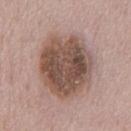Impression: No biopsy was performed on this lesion — it was imaged during a full skin examination and was not determined to be concerning. Context: On the chest. The total-body-photography lesion software estimated an average lesion color of about L≈50 a*≈17 b*≈24 (CIELAB) and a lesion-to-skin contrast of about 10 (normalized; higher = more distinct). The analysis additionally found a border-irregularity index near 1.5/10 and a within-lesion color-variation index near 6/10. The software also gave a nevus-likeness score of about 5/100 and a detector confidence of about 100 out of 100 that the crop contains a lesion. A male subject, aged approximately 50. The recorded lesion diameter is about 7.5 mm. Cropped from a whole-body photographic skin survey; the tile spans about 15 mm.